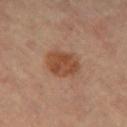This lesion was catalogued during total-body skin photography and was not selected for biopsy.